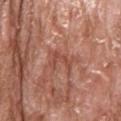notes = catalogued during a skin exam; not biopsied
body site = the head or neck
image source = ~15 mm tile from a whole-body skin photo
patient = male, approximately 65 years of age